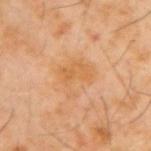Case summary:
– biopsy status: catalogued during a skin exam; not biopsied
– lighting: cross-polarized
– subject: male, aged 58–62
– site: the upper back
– image: 15 mm crop, total-body photography